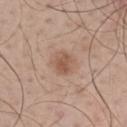Assessment:
No biopsy was performed on this lesion — it was imaged during a full skin examination and was not determined to be concerning.
Acquisition and patient details:
The lesion is on the upper back. The patient is a male aged around 45. The lesion-visualizer software estimated internal color variation of about 2.5 on a 0–10 scale and a peripheral color-asymmetry measure near 1. Measured at roughly 3 mm in maximum diameter. The tile uses white-light illumination. A region of skin cropped from a whole-body photographic capture, roughly 15 mm wide.